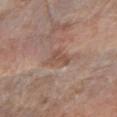  biopsy_status: not biopsied; imaged during a skin examination
  lesion_size:
    long_diameter_mm_approx: 3.0
  patient:
    sex: female
    age_approx: 65
  site: arm
  image:
    source: total-body photography crop
    field_of_view_mm: 15